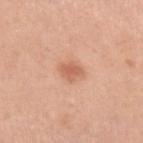anatomic site = the left upper arm
patient = female, aged around 55
size = ≈2.5 mm
tile lighting = white-light
image source = 15 mm crop, total-body photography